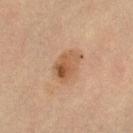Part of a total-body skin-imaging series; this lesion was reviewed on a skin check and was not flagged for biopsy.
The subject is a female about 30 years old.
A lesion tile, about 15 mm wide, cut from a 3D total-body photograph.
On the leg.